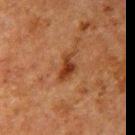* workup: total-body-photography surveillance lesion; no biopsy
* size: ≈3.5 mm
* anatomic site: the right upper arm
* image: 15 mm crop, total-body photography
* patient: male, roughly 60 years of age